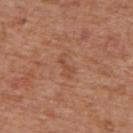biopsy status: no biopsy performed (imaged during a skin exam)
body site: the upper back
image-analysis metrics: a footprint of about 3 mm², an outline eccentricity of about 0.9 (0 = round, 1 = elongated), and a symmetry-axis asymmetry near 0.45; a lesion color around L≈49 a*≈24 b*≈32 in CIELAB, roughly 6 lightness units darker than nearby skin, and a normalized border contrast of about 5; an automated nevus-likeness rating near 0 out of 100 and lesion-presence confidence of about 100/100
tile lighting: white-light illumination
subject: male, aged around 65
acquisition: ~15 mm crop, total-body skin-cancer survey
lesion size: about 3 mm